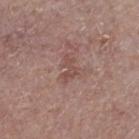automated lesion analysis: a footprint of about 3.5 mm², an eccentricity of roughly 0.85, and a shape-asymmetry score of about 0.6 (0 = symmetric); a classifier nevus-likeness of about 0/100 and lesion-presence confidence of about 100/100 | illumination: white-light | lesion size: ~3.5 mm (longest diameter) | site: the left leg | subject: male, in their 80s | image: ~15 mm crop, total-body skin-cancer survey.